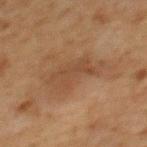Impression: The lesion was photographed on a routine skin check and not biopsied; there is no pathology result. Clinical summary: The total-body-photography lesion software estimated roughly 5 lightness units darker than nearby skin and a normalized lesion–skin contrast near 5. The analysis additionally found a border-irregularity index near 7.5/10 and a peripheral color-asymmetry measure near 0.5. It also reported a classifier nevus-likeness of about 0/100 and lesion-presence confidence of about 95/100. From the back. Approximately 8.5 mm at its widest. A close-up tile cropped from a whole-body skin photograph, about 15 mm across. The subject is a female about 60 years old. The tile uses cross-polarized illumination.